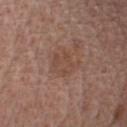notes — catalogued during a skin exam; not biopsied | lesion size — ≈3.5 mm | subject — male, in their mid-50s | location — the chest | lighting — white-light illumination | acquisition — total-body-photography crop, ~15 mm field of view.